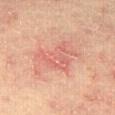The lesion was photographed on a routine skin check and not biopsied; there is no pathology result.